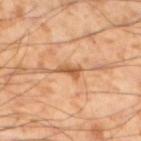{"biopsy_status": "not biopsied; imaged during a skin examination", "patient": {"sex": "male", "age_approx": 55}, "image": {"source": "total-body photography crop", "field_of_view_mm": 15}, "site": "left lower leg"}